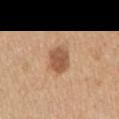Case summary:
– biopsy status — imaged on a skin check; not biopsied
– site — the right upper arm
– imaging modality — ~15 mm tile from a whole-body skin photo
– patient — female, roughly 40 years of age